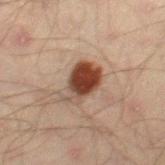Part of a total-body skin-imaging series; this lesion was reviewed on a skin check and was not flagged for biopsy.
A 15 mm crop from a total-body photograph taken for skin-cancer surveillance.
A male subject, aged 43–47.
The tile uses cross-polarized illumination.
From the right thigh.